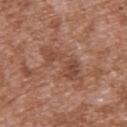About 6 mm across.
A male patient, aged approximately 45.
A 15 mm close-up tile from a total-body photography series done for melanoma screening.
The lesion is on the upper back.